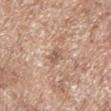Imaged during a routine full-body skin examination; the lesion was not biopsied and no histopathology is available.
A 15 mm crop from a total-body photograph taken for skin-cancer surveillance.
The subject is a male aged 68–72.
Imaged with white-light lighting.
Located on the left upper arm.
Longest diameter approximately 2.5 mm.